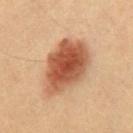{"image": {"source": "total-body photography crop", "field_of_view_mm": 15}, "site": "chest", "patient": {"sex": "male", "age_approx": 60}}